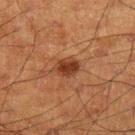Findings:
- anatomic site — the arm
- acquisition — ~15 mm tile from a whole-body skin photo
- subject — male, aged approximately 60